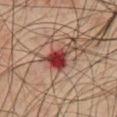From the chest.
Automated image analysis of the tile measured an area of roughly 7 mm², an outline eccentricity of about 0.65 (0 = round, 1 = elongated), and two-axis asymmetry of about 0.45. And it measured a nevus-likeness score of about 0/100 and a lesion-detection confidence of about 100/100.
Approximately 4 mm at its widest.
Imaged with cross-polarized lighting.
A lesion tile, about 15 mm wide, cut from a 3D total-body photograph.
A male subject aged 73 to 77.